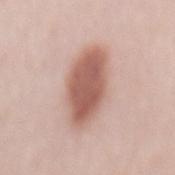biopsy_status: not biopsied; imaged during a skin examination
patient:
  sex: female
  age_approx: 55
site: mid back
lighting: white-light
image:
  source: total-body photography crop
  field_of_view_mm: 15